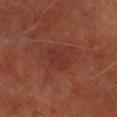The lesion was photographed on a routine skin check and not biopsied; there is no pathology result. The lesion's longest dimension is about 3.5 mm. On the right lower leg. Cropped from a whole-body photographic skin survey; the tile spans about 15 mm. A male patient, aged 68 to 72. The total-body-photography lesion software estimated an average lesion color of about L≈26 a*≈23 b*≈21 (CIELAB), a lesion–skin lightness drop of about 4, and a normalized border contrast of about 5. It also reported a detector confidence of about 100 out of 100 that the crop contains a lesion.